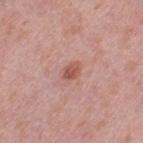This lesion was catalogued during total-body skin photography and was not selected for biopsy.
The lesion's longest dimension is about 2.5 mm.
An algorithmic analysis of the crop reported a lesion area of about 3 mm². And it measured a border-irregularity rating of about 1.5/10, a color-variation rating of about 2.5/10, and radial color variation of about 1. It also reported an automated nevus-likeness rating near 65 out of 100.
The lesion is located on the left thigh.
A 15 mm close-up extracted from a 3D total-body photography capture.
A female subject approximately 40 years of age.
The tile uses white-light illumination.